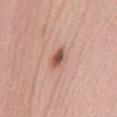Assessment: Imaged during a routine full-body skin examination; the lesion was not biopsied and no histopathology is available. Background: A female patient aged 38 to 42. Longest diameter approximately 2.5 mm. Captured under white-light illumination. A 15 mm crop from a total-body photograph taken for skin-cancer surveillance. From the mid back.